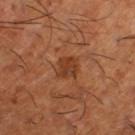diameter — ≈2.5 mm; patient — male, aged approximately 65; site — the right lower leg; image source — 15 mm crop, total-body photography.